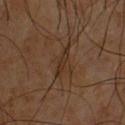<lesion>
  <biopsy_status>not biopsied; imaged during a skin examination</biopsy_status>
  <image>
    <source>total-body photography crop</source>
    <field_of_view_mm>15</field_of_view_mm>
  </image>
  <automated_metrics>
    <area_mm2_approx>3.0</area_mm2_approx>
    <eccentricity>0.95</eccentricity>
    <shape_asymmetry>0.3</shape_asymmetry>
    <cielab_L>27</cielab_L>
    <cielab_a>15</cielab_a>
    <cielab_b>24</cielab_b>
    <vs_skin_darker_L>6.0</vs_skin_darker_L>
    <vs_skin_contrast_norm>7.0</vs_skin_contrast_norm>
    <border_irregularity_0_10>4.5</border_irregularity_0_10>
    <peripheral_color_asymmetry>0.0</peripheral_color_asymmetry>
    <lesion_detection_confidence_0_100>55</lesion_detection_confidence_0_100>
  </automated_metrics>
  <lighting>cross-polarized</lighting>
  <site>chest</site>
  <lesion_size>
    <long_diameter_mm_approx>3.5</long_diameter_mm_approx>
  </lesion_size>
  <patient>
    <sex>male</sex>
    <age_approx>60</age_approx>
  </patient>
</lesion>